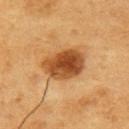follow-up: catalogued during a skin exam; not biopsied | image: total-body-photography crop, ~15 mm field of view | site: the right upper arm | subject: male, in their 60s | illumination: cross-polarized.